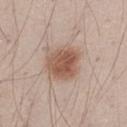Notes:
- workup — catalogued during a skin exam; not biopsied
- subject — male, approximately 30 years of age
- size — ~4.5 mm (longest diameter)
- location — the left thigh
- imaging modality — ~15 mm crop, total-body skin-cancer survey
- illumination — white-light